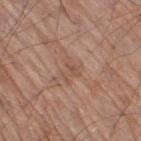Findings:
– notes: imaged on a skin check; not biopsied
– subject: male, aged 68 to 72
– lesion diameter: ≈3 mm
– lighting: white-light
– acquisition: total-body-photography crop, ~15 mm field of view
– automated metrics: an average lesion color of about L≈52 a*≈19 b*≈29 (CIELAB), a lesion–skin lightness drop of about 6, and a normalized border contrast of about 5; a border-irregularity rating of about 7.5/10, internal color variation of about 2 on a 0–10 scale, and radial color variation of about 1
– body site: the right thigh